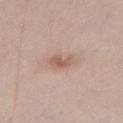Q: Was this lesion biopsied?
A: catalogued during a skin exam; not biopsied
Q: Who is the patient?
A: male, aged around 55
Q: Where on the body is the lesion?
A: the abdomen
Q: What kind of image is this?
A: total-body-photography crop, ~15 mm field of view